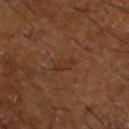Q: Is there a histopathology result?
A: no biopsy performed (imaged during a skin exam)
Q: How was the tile lit?
A: cross-polarized illumination
Q: What is the anatomic site?
A: the left lower leg
Q: What is the imaging modality?
A: ~15 mm tile from a whole-body skin photo
Q: Automated lesion metrics?
A: an eccentricity of roughly 0.9 and a symmetry-axis asymmetry near 0.3
Q: What are the patient's age and sex?
A: male, in their mid-60s